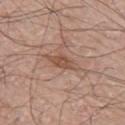Impression: This lesion was catalogued during total-body skin photography and was not selected for biopsy. Context: Imaged with white-light lighting. On the left arm. The patient is a male about 70 years old. Measured at roughly 4.5 mm in maximum diameter. A 15 mm close-up extracted from a 3D total-body photography capture. Automated image analysis of the tile measured a footprint of about 6 mm², a shape eccentricity near 0.9, and a shape-asymmetry score of about 0.2 (0 = symmetric). The analysis additionally found a lesion–skin lightness drop of about 9 and a normalized lesion–skin contrast near 7.